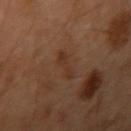This lesion was catalogued during total-body skin photography and was not selected for biopsy.